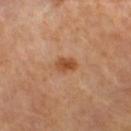This lesion was catalogued during total-body skin photography and was not selected for biopsy. The lesion's longest dimension is about 3 mm. A 15 mm close-up tile from a total-body photography series done for melanoma screening. The subject is aged around 70. On the right thigh. This is a cross-polarized tile.